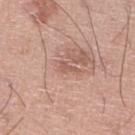The patient is a male about 20 years old. Approximately 2 mm at its widest. The lesion is on the right lower leg. Captured under white-light illumination. Cropped from a total-body skin-imaging series; the visible field is about 15 mm.